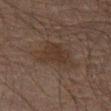• notes — imaged on a skin check; not biopsied
• anatomic site — the left thigh
• subject — male, aged 58 to 62
• image-analysis metrics — a nevus-likeness score of about 15/100
• illumination — cross-polarized illumination
• image source — 15 mm crop, total-body photography
• lesion diameter — ≈6 mm